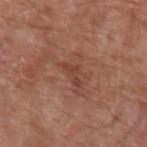Q: Was a biopsy performed?
A: imaged on a skin check; not biopsied
Q: Lesion size?
A: about 3 mm
Q: What is the imaging modality?
A: 15 mm crop, total-body photography
Q: What are the patient's age and sex?
A: male, aged 78–82
Q: Automated lesion metrics?
A: a lesion area of about 2.5 mm² and a symmetry-axis asymmetry near 0.6
Q: Lesion location?
A: the right forearm